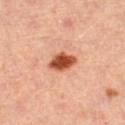| feature | finding |
|---|---|
| image source | 15 mm crop, total-body photography |
| site | the left thigh |
| subject | female, roughly 45 years of age |